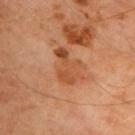Clinical impression:
Imaged during a routine full-body skin examination; the lesion was not biopsied and no histopathology is available.
Clinical summary:
The lesion is on the back. A 15 mm crop from a total-body photograph taken for skin-cancer surveillance. Captured under cross-polarized illumination. The recorded lesion diameter is about 4.5 mm. A male subject, roughly 65 years of age.